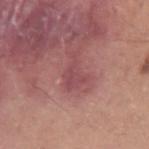Imaged during a routine full-body skin examination; the lesion was not biopsied and no histopathology is available. Automated image analysis of the tile measured a mean CIELAB color near L≈48 a*≈28 b*≈19, about 7 CIELAB-L* units darker than the surrounding skin, and a normalized border contrast of about 5.5. And it measured border irregularity of about 7 on a 0–10 scale and internal color variation of about 1.5 on a 0–10 scale. The analysis additionally found a classifier nevus-likeness of about 0/100 and a lesion-detection confidence of about 65/100. Imaged with white-light lighting. The recorded lesion diameter is about 4 mm. A male patient, aged 28 to 32. A roughly 15 mm field-of-view crop from a total-body skin photograph. On the left upper arm.